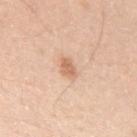body site = the left upper arm | lighting = white-light illumination | image source = total-body-photography crop, ~15 mm field of view | subject = male, about 30 years old | lesion size = ~2.5 mm (longest diameter).